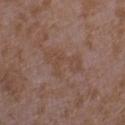From the left forearm.
Longest diameter approximately 5 mm.
Automated image analysis of the tile measured a footprint of about 8.5 mm² and two-axis asymmetry of about 0.5. And it measured a lesion color around L≈45 a*≈17 b*≈26 in CIELAB, about 5 CIELAB-L* units darker than the surrounding skin, and a normalized lesion–skin contrast near 5. And it measured a color-variation rating of about 2.5/10 and a peripheral color-asymmetry measure near 1. And it measured a classifier nevus-likeness of about 0/100 and a lesion-detection confidence of about 100/100.
A female subject, aged approximately 35.
A 15 mm crop from a total-body photograph taken for skin-cancer surveillance.
Captured under white-light illumination.